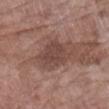Q: What did automated image analysis measure?
A: a lesion area of about 10 mm², a shape eccentricity near 0.6, and a shape-asymmetry score of about 0.25 (0 = symmetric); a border-irregularity rating of about 2.5/10 and a color-variation rating of about 2/10; a classifier nevus-likeness of about 0/100 and a lesion-detection confidence of about 100/100
Q: What are the patient's age and sex?
A: female, in their 70s
Q: What is the anatomic site?
A: the left forearm
Q: What kind of image is this?
A: 15 mm crop, total-body photography
Q: How was the tile lit?
A: white-light
Q: How large is the lesion?
A: ≈4 mm